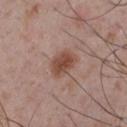Imaged during a routine full-body skin examination; the lesion was not biopsied and no histopathology is available. Automated tile analysis of the lesion measured an area of roughly 7.5 mm², a shape eccentricity near 0.75, and a symmetry-axis asymmetry near 0.15. It also reported a lesion color around L≈47 a*≈21 b*≈26 in CIELAB, about 11 CIELAB-L* units darker than the surrounding skin, and a normalized border contrast of about 8.5. It also reported a nevus-likeness score of about 90/100 and a lesion-detection confidence of about 100/100. Longest diameter approximately 3.5 mm. A 15 mm close-up tile from a total-body photography series done for melanoma screening. A male subject, aged around 55. The lesion is on the chest. The tile uses white-light illumination.